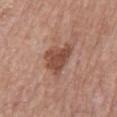A lesion tile, about 15 mm wide, cut from a 3D total-body photograph. A male patient, aged approximately 80. About 4.5 mm across. On the mid back.